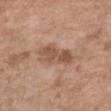Captured during whole-body skin photography for melanoma surveillance; the lesion was not biopsied. Imaged with white-light lighting. The total-body-photography lesion software estimated a lesion area of about 11 mm², a shape eccentricity near 0.9, and a symmetry-axis asymmetry near 0.25. The analysis additionally found a normalized lesion–skin contrast near 7. And it measured an automated nevus-likeness rating near 0 out of 100 and lesion-presence confidence of about 100/100. A female patient aged 73–77. A region of skin cropped from a whole-body photographic capture, roughly 15 mm wide. On the arm.